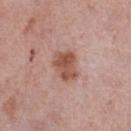The lesion was tiled from a total-body skin photograph and was not biopsied. The lesion is located on the chest. A male patient aged 73 to 77. A 15 mm close-up extracted from a 3D total-body photography capture. This is a white-light tile. Approximately 3.5 mm at its widest.